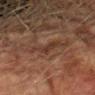The lesion was photographed on a routine skin check and not biopsied; there is no pathology result.
On the left forearm.
The lesion's longest dimension is about 3 mm.
Cropped from a total-body skin-imaging series; the visible field is about 15 mm.
A male patient in their mid- to late 70s.